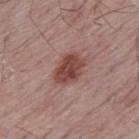follow-up = imaged on a skin check; not biopsied
illumination = white-light
subject = male, approximately 65 years of age
imaging modality = ~15 mm crop, total-body skin-cancer survey
image-analysis metrics = border irregularity of about 1.5 on a 0–10 scale, a within-lesion color-variation index near 4/10, and radial color variation of about 1.5; a classifier nevus-likeness of about 100/100 and a lesion-detection confidence of about 100/100
location = the back